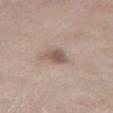Impression: The lesion was tiled from a total-body skin photograph and was not biopsied. Image and clinical context: On the abdomen. The lesion-visualizer software estimated an area of roughly 5 mm², an eccentricity of roughly 0.6, and a shape-asymmetry score of about 0.25 (0 = symmetric). And it measured a classifier nevus-likeness of about 45/100 and a lesion-detection confidence of about 100/100. The subject is a female in their mid- to late 70s. Captured under white-light illumination. Longest diameter approximately 3 mm. A lesion tile, about 15 mm wide, cut from a 3D total-body photograph.